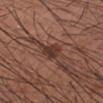Q: Was a biopsy performed?
A: total-body-photography surveillance lesion; no biopsy
Q: What lighting was used for the tile?
A: white-light
Q: What is the anatomic site?
A: the right forearm
Q: What kind of image is this?
A: ~15 mm crop, total-body skin-cancer survey
Q: Patient demographics?
A: male, roughly 30 years of age